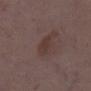Imaged during a routine full-body skin examination; the lesion was not biopsied and no histopathology is available.
The subject is a female aged around 35.
The total-body-photography lesion software estimated an area of roughly 4 mm², an outline eccentricity of about 0.8 (0 = round, 1 = elongated), and a symmetry-axis asymmetry near 0.35. The software also gave an automated nevus-likeness rating near 0 out of 100 and a lesion-detection confidence of about 100/100.
A roughly 15 mm field-of-view crop from a total-body skin photograph.
Approximately 3 mm at its widest.
The lesion is on the left lower leg.
Imaged with white-light lighting.